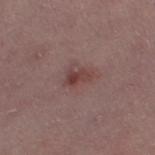The lesion was photographed on a routine skin check and not biopsied; there is no pathology result. A female patient, in their mid- to late 40s. Cropped from a total-body skin-imaging series; the visible field is about 15 mm. The lesion is on the left thigh.